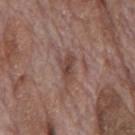{"biopsy_status": "not biopsied; imaged during a skin examination", "site": "mid back", "patient": {"sex": "male", "age_approx": 70}, "lesion_size": {"long_diameter_mm_approx": 3.0}, "image": {"source": "total-body photography crop", "field_of_view_mm": 15}}